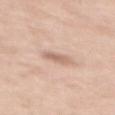Part of a total-body skin-imaging series; this lesion was reviewed on a skin check and was not flagged for biopsy.
A lesion tile, about 15 mm wide, cut from a 3D total-body photograph.
The lesion is located on the upper back.
The patient is a female aged 53–57.
The lesion's longest dimension is about 2.5 mm.